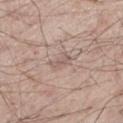Part of a total-body skin-imaging series; this lesion was reviewed on a skin check and was not flagged for biopsy. The total-body-photography lesion software estimated a footprint of about 2.5 mm², an eccentricity of roughly 0.9, and a symmetry-axis asymmetry near 0.35. It also reported a border-irregularity index near 3.5/10, internal color variation of about 0 on a 0–10 scale, and peripheral color asymmetry of about 0. And it measured a classifier nevus-likeness of about 0/100. A lesion tile, about 15 mm wide, cut from a 3D total-body photograph. Captured under white-light illumination. A male subject, aged around 55. The lesion is on the left thigh.